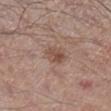Notes:
* notes · imaged on a skin check; not biopsied
* image · total-body-photography crop, ~15 mm field of view
* site · the leg
* subject · male, aged approximately 55